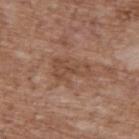{
  "biopsy_status": "not biopsied; imaged during a skin examination",
  "image": {
    "source": "total-body photography crop",
    "field_of_view_mm": 15
  },
  "site": "upper back",
  "patient": {
    "sex": "male",
    "age_approx": 70
  },
  "automated_metrics": {
    "border_irregularity_0_10": 7.0,
    "color_variation_0_10": 2.0,
    "peripheral_color_asymmetry": 0.5
  },
  "lesion_size": {
    "long_diameter_mm_approx": 4.5
  }
}